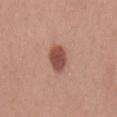workup: total-body-photography surveillance lesion; no biopsy
body site: the back
lighting: white-light illumination
image source: total-body-photography crop, ~15 mm field of view
lesion size: about 3.5 mm
patient: male, aged around 30
image-analysis metrics: an outline eccentricity of about 0.75 (0 = round, 1 = elongated) and a symmetry-axis asymmetry near 0.15; a lesion color around L≈50 a*≈23 b*≈27 in CIELAB, a lesion–skin lightness drop of about 14, and a lesion-to-skin contrast of about 9.5 (normalized; higher = more distinct); a border-irregularity index near 1.5/10, internal color variation of about 2.5 on a 0–10 scale, and radial color variation of about 0.5